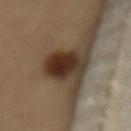Findings:
• biopsy status: total-body-photography surveillance lesion; no biopsy
• lighting: cross-polarized illumination
• patient: female, approximately 70 years of age
• body site: the mid back
• TBP lesion metrics: an area of roughly 13 mm², an eccentricity of roughly 0.55, and a shape-asymmetry score of about 0.15 (0 = symmetric); a nevus-likeness score of about 100/100 and a lesion-detection confidence of about 100/100
• diameter: ~4.5 mm (longest diameter)
• imaging modality: 15 mm crop, total-body photography